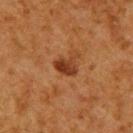<tbp_lesion>
  <biopsy_status>not biopsied; imaged during a skin examination</biopsy_status>
  <patient>
    <sex>male</sex>
    <age_approx>60</age_approx>
  </patient>
  <lesion_size>
    <long_diameter_mm_approx>3.0</long_diameter_mm_approx>
  </lesion_size>
  <image>
    <source>total-body photography crop</source>
    <field_of_view_mm>15</field_of_view_mm>
  </image>
  <site>upper back</site>
  <lighting>cross-polarized</lighting>
  <automated_metrics>
    <area_mm2_approx>5.0</area_mm2_approx>
    <eccentricity>0.65</eccentricity>
    <shape_asymmetry>0.3</shape_asymmetry>
    <vs_skin_darker_L>10.0</vs_skin_darker_L>
    <nevus_likeness_0_100>85</nevus_likeness_0_100>
    <lesion_detection_confidence_0_100>100</lesion_detection_confidence_0_100>
  </automated_metrics>
</tbp_lesion>